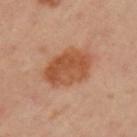Imaged during a routine full-body skin examination; the lesion was not biopsied and no histopathology is available. A female patient about 35 years old. Captured under cross-polarized illumination. Located on the left upper arm. A 15 mm close-up extracted from a 3D total-body photography capture.